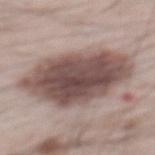{"lesion_size": {"long_diameter_mm_approx": 10.0}, "image": {"source": "total-body photography crop", "field_of_view_mm": 15}, "site": "back", "automated_metrics": {"area_mm2_approx": 43.0, "eccentricity": 0.8, "shape_asymmetry": 0.15, "border_irregularity_0_10": 2.5}, "patient": {"sex": "male", "age_approx": 65}}